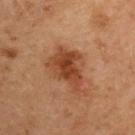- notes: no biopsy performed (imaged during a skin exam)
- size: ≈5.5 mm
- lighting: cross-polarized
- automated lesion analysis: a nevus-likeness score of about 60/100 and a detector confidence of about 100 out of 100 that the crop contains a lesion
- subject: male, approximately 70 years of age
- anatomic site: the right upper arm
- image: ~15 mm crop, total-body skin-cancer survey